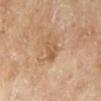{"biopsy_status": "not biopsied; imaged during a skin examination", "image": {"source": "total-body photography crop", "field_of_view_mm": 15}, "site": "left lower leg", "automated_metrics": {"cielab_L": 57, "cielab_a": 20, "cielab_b": 36, "vs_skin_darker_L": 8.0, "vs_skin_contrast_norm": 5.5, "border_irregularity_0_10": 3.5, "color_variation_0_10": 3.5, "peripheral_color_asymmetry": 1.0}, "lesion_size": {"long_diameter_mm_approx": 3.0}, "lighting": "cross-polarized", "patient": {"sex": "male", "age_approx": 65}}